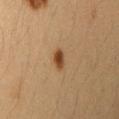The lesion was tiled from a total-body skin photograph and was not biopsied.
On the arm.
A female subject, aged 28 to 32.
Automated image analysis of the tile measured a mean CIELAB color near L≈41 a*≈19 b*≈33 and roughly 12 lightness units darker than nearby skin. It also reported a border-irregularity index near 2/10, a color-variation rating of about 3/10, and peripheral color asymmetry of about 0.5. And it measured a nevus-likeness score of about 100/100 and a lesion-detection confidence of about 100/100.
Cropped from a whole-body photographic skin survey; the tile spans about 15 mm.